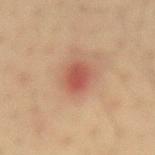<record>
  <biopsy_status>not biopsied; imaged during a skin examination</biopsy_status>
  <patient>
    <sex>male</sex>
    <age_approx>35</age_approx>
  </patient>
  <site>back</site>
  <image>
    <source>total-body photography crop</source>
    <field_of_view_mm>15</field_of_view_mm>
  </image>
</record>